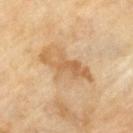Q: Was a biopsy performed?
A: total-body-photography surveillance lesion; no biopsy
Q: Who is the patient?
A: male, aged 63 to 67
Q: What is the imaging modality?
A: ~15 mm crop, total-body skin-cancer survey